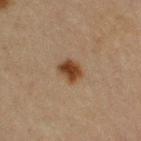Captured during whole-body skin photography for melanoma surveillance; the lesion was not biopsied. A close-up tile cropped from a whole-body skin photograph, about 15 mm across. The tile uses cross-polarized illumination. A female subject, approximately 65 years of age. From the right upper arm. About 3 mm across.